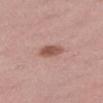Part of a total-body skin-imaging series; this lesion was reviewed on a skin check and was not flagged for biopsy.
The lesion is located on the right thigh.
Longest diameter approximately 3 mm.
A lesion tile, about 15 mm wide, cut from a 3D total-body photograph.
Captured under white-light illumination.
The patient is a female about 45 years old.